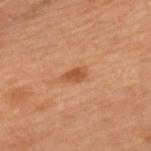Q: Was a biopsy performed?
A: imaged on a skin check; not biopsied
Q: What is the imaging modality?
A: total-body-photography crop, ~15 mm field of view
Q: How large is the lesion?
A: ≈2.5 mm
Q: Patient demographics?
A: male, in their 50s
Q: Lesion location?
A: the chest